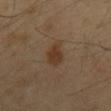{
  "biopsy_status": "not biopsied; imaged during a skin examination",
  "lesion_size": {
    "long_diameter_mm_approx": 3.5
  },
  "lighting": "cross-polarized",
  "site": "mid back",
  "patient": {
    "sex": "male",
    "age_approx": 40
  },
  "automated_metrics": {
    "cielab_L": 35,
    "cielab_a": 16,
    "cielab_b": 28,
    "vs_skin_darker_L": 8.0,
    "vs_skin_contrast_norm": 8.0,
    "border_irregularity_0_10": 2.5,
    "color_variation_0_10": 2.5,
    "peripheral_color_asymmetry": 1.0,
    "nevus_likeness_0_100": 90,
    "lesion_detection_confidence_0_100": 100
  },
  "image": {
    "source": "total-body photography crop",
    "field_of_view_mm": 15
  }
}